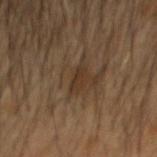Assessment: Imaged during a routine full-body skin examination; the lesion was not biopsied and no histopathology is available. Context: This is a cross-polarized tile. Measured at roughly 2.5 mm in maximum diameter. The lesion-visualizer software estimated a lesion area of about 2.5 mm², an outline eccentricity of about 0.9 (0 = round, 1 = elongated), and a symmetry-axis asymmetry near 0.35. The software also gave an average lesion color of about L≈29 a*≈13 b*≈25 (CIELAB), a lesion–skin lightness drop of about 6, and a normalized border contrast of about 6.5. The analysis additionally found border irregularity of about 3.5 on a 0–10 scale, a within-lesion color-variation index near 0/10, and radial color variation of about 0. The analysis additionally found a lesion-detection confidence of about 85/100. On the head or neck. The subject is a male in their mid- to late 50s. A lesion tile, about 15 mm wide, cut from a 3D total-body photograph.